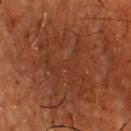Clinical impression:
Captured during whole-body skin photography for melanoma surveillance; the lesion was not biopsied.
Context:
The subject is a male roughly 55 years of age. The lesion is located on the front of the torso. Cropped from a whole-body photographic skin survey; the tile spans about 15 mm.